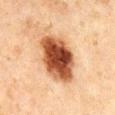| feature | finding |
|---|---|
| notes | catalogued during a skin exam; not biopsied |
| subject | male, in their mid- to late 40s |
| lesion size | about 6.5 mm |
| illumination | cross-polarized |
| body site | the mid back |
| image | total-body-photography crop, ~15 mm field of view |